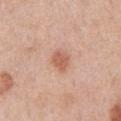Q: Is there a histopathology result?
A: total-body-photography surveillance lesion; no biopsy
Q: What are the patient's age and sex?
A: male, roughly 70 years of age
Q: What did automated image analysis measure?
A: an outline eccentricity of about 0.6 (0 = round, 1 = elongated) and two-axis asymmetry of about 0.2; a color-variation rating of about 2/10 and radial color variation of about 0.5; an automated nevus-likeness rating near 90 out of 100 and lesion-presence confidence of about 100/100
Q: Illumination type?
A: white-light
Q: What is the imaging modality?
A: total-body-photography crop, ~15 mm field of view
Q: What is the anatomic site?
A: the abdomen